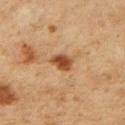Impression: Captured during whole-body skin photography for melanoma surveillance; the lesion was not biopsied. Clinical summary: Imaged with cross-polarized lighting. The lesion-visualizer software estimated roughly 13 lightness units darker than nearby skin and a normalized lesion–skin contrast near 10.5. Located on the right upper arm. Cropped from a total-body skin-imaging series; the visible field is about 15 mm. A female subject, about 45 years old. The lesion's longest dimension is about 3 mm.